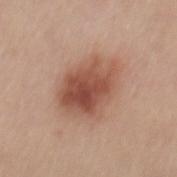Case summary:
* notes — catalogued during a skin exam; not biopsied
* body site — the mid back
* image — ~15 mm crop, total-body skin-cancer survey
* subject — male, in their 30s
* TBP lesion metrics — a border-irregularity index near 3/10, a color-variation rating of about 6.5/10, and peripheral color asymmetry of about 2
* lighting — white-light illumination
* lesion diameter — ~6 mm (longest diameter)